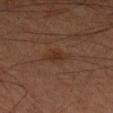Clinical impression: The lesion was photographed on a routine skin check and not biopsied; there is no pathology result. Image and clinical context: A region of skin cropped from a whole-body photographic capture, roughly 15 mm wide. A male patient in their 60s. From the right forearm. About 3.5 mm across. Automated image analysis of the tile measured an area of roughly 5.5 mm² and an eccentricity of roughly 0.75. And it measured a mean CIELAB color near L≈28 a*≈17 b*≈25 and a lesion–skin lightness drop of about 5. The analysis additionally found an automated nevus-likeness rating near 45 out of 100 and lesion-presence confidence of about 100/100. The tile uses cross-polarized illumination.